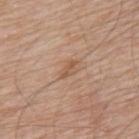Impression: Recorded during total-body skin imaging; not selected for excision or biopsy. Acquisition and patient details: Automated tile analysis of the lesion measured a footprint of about 2.5 mm², an eccentricity of roughly 0.85, and a symmetry-axis asymmetry near 0.3. The analysis additionally found a color-variation rating of about 1/10 and a peripheral color-asymmetry measure near 0.5. It also reported an automated nevus-likeness rating near 0 out of 100 and a lesion-detection confidence of about 100/100. This is a white-light tile. The lesion is located on the upper back. A male subject, about 80 years old. A 15 mm crop from a total-body photograph taken for skin-cancer surveillance. The recorded lesion diameter is about 2.5 mm.